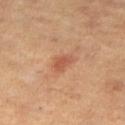biopsy_status: not biopsied; imaged during a skin examination
image:
  source: total-body photography crop
  field_of_view_mm: 15
patient:
  sex: female
  age_approx: 40
site: leg